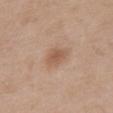Clinical impression:
The lesion was tiled from a total-body skin photograph and was not biopsied.
Context:
Cropped from a whole-body photographic skin survey; the tile spans about 15 mm. On the upper back. About 3 mm across. The tile uses white-light illumination. A female patient, aged 58 to 62.